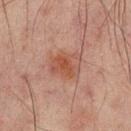No biopsy was performed on this lesion — it was imaged during a full skin examination and was not determined to be concerning.
Located on the mid back.
Cropped from a total-body skin-imaging series; the visible field is about 15 mm.
Captured under cross-polarized illumination.
Automated image analysis of the tile measured about 7 CIELAB-L* units darker than the surrounding skin and a lesion-to-skin contrast of about 6.5 (normalized; higher = more distinct).
A male subject, approximately 65 years of age.
Approximately 4 mm at its widest.